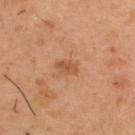notes: no biopsy performed (imaged during a skin exam) | anatomic site: the upper back | patient: male, aged approximately 55 | tile lighting: cross-polarized | size: ≈2.5 mm | image source: 15 mm crop, total-body photography | TBP lesion metrics: a mean CIELAB color near L≈51 a*≈23 b*≈37, a lesion–skin lightness drop of about 8, and a normalized border contrast of about 6.5; a classifier nevus-likeness of about 10/100.